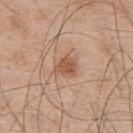A lesion tile, about 15 mm wide, cut from a 3D total-body photograph.
This is a white-light tile.
About 3 mm across.
A male patient, roughly 55 years of age.
From the upper back.